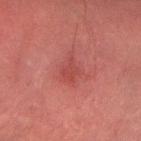The lesion is located on the right forearm.
The lesion-visualizer software estimated internal color variation of about 1 on a 0–10 scale and peripheral color asymmetry of about 0.5. The software also gave a classifier nevus-likeness of about 0/100 and a detector confidence of about 85 out of 100 that the crop contains a lesion.
This image is a 15 mm lesion crop taken from a total-body photograph.
A male patient, in their 70s.